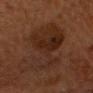Captured during whole-body skin photography for melanoma surveillance; the lesion was not biopsied. A male patient in their mid- to late 50s. The lesion is located on the head or neck. The lesion-visualizer software estimated an average lesion color of about L≈23 a*≈17 b*≈24 (CIELAB). The analysis additionally found internal color variation of about 4 on a 0–10 scale. Longest diameter approximately 8 mm. Captured under cross-polarized illumination. A 15 mm close-up extracted from a 3D total-body photography capture.